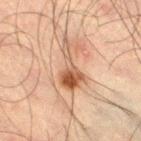Assessment: The lesion was photographed on a routine skin check and not biopsied; there is no pathology result. Context: A male subject, aged around 50. A region of skin cropped from a whole-body photographic capture, roughly 15 mm wide. Imaged with cross-polarized lighting. On the right thigh. Approximately 6 mm at its widest.